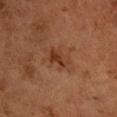Impression:
Recorded during total-body skin imaging; not selected for excision or biopsy.
Background:
The lesion is on the left upper arm. A female subject, about 60 years old. The tile uses cross-polarized illumination. About 3.5 mm across. Cropped from a whole-body photographic skin survey; the tile spans about 15 mm. The total-body-photography lesion software estimated an area of roughly 5 mm², an outline eccentricity of about 0.85 (0 = round, 1 = elongated), and a symmetry-axis asymmetry near 0.35. It also reported a border-irregularity rating of about 4/10 and a peripheral color-asymmetry measure near 0.5. The analysis additionally found lesion-presence confidence of about 100/100.